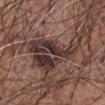No biopsy was performed on this lesion — it was imaged during a full skin examination and was not determined to be concerning.
Measured at roughly 8.5 mm in maximum diameter.
A lesion tile, about 15 mm wide, cut from a 3D total-body photograph.
From the arm.
This is a white-light tile.
A male patient, aged approximately 60.